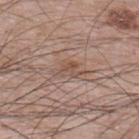notes — catalogued during a skin exam; not biopsied | lighting — white-light illumination | subject — male, approximately 65 years of age | site — the upper back | image-analysis metrics — a lesion color around L≈51 a*≈17 b*≈26 in CIELAB, roughly 8 lightness units darker than nearby skin, and a lesion-to-skin contrast of about 6 (normalized; higher = more distinct); a within-lesion color-variation index near 2/10; a nevus-likeness score of about 0/100 and lesion-presence confidence of about 70/100 | acquisition — ~15 mm crop, total-body skin-cancer survey | lesion size — about 3.5 mm.